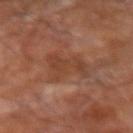Q: Lesion location?
A: the right forearm
Q: What kind of image is this?
A: ~15 mm crop, total-body skin-cancer survey
Q: Illumination type?
A: cross-polarized
Q: What are the patient's age and sex?
A: male, roughly 65 years of age
Q: What did automated image analysis measure?
A: a lesion area of about 10 mm² and a shape-asymmetry score of about 0.5 (0 = symmetric); a detector confidence of about 100 out of 100 that the crop contains a lesion
Q: What is the lesion's diameter?
A: about 5.5 mm